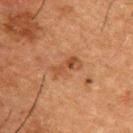No biopsy was performed on this lesion — it was imaged during a full skin examination and was not determined to be concerning. About 3.5 mm across. A male subject, in their 50s. Automated image analysis of the tile measured border irregularity of about 4 on a 0–10 scale, a color-variation rating of about 4.5/10, and radial color variation of about 1.5. From the upper back. A 15 mm close-up tile from a total-body photography series done for melanoma screening.